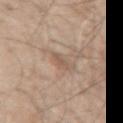Q: Was this lesion biopsied?
A: total-body-photography surveillance lesion; no biopsy
Q: What are the patient's age and sex?
A: male, aged around 50
Q: What kind of image is this?
A: ~15 mm tile from a whole-body skin photo
Q: Lesion location?
A: the right upper arm
Q: How large is the lesion?
A: about 2.5 mm
Q: Illumination type?
A: white-light
Q: Automated lesion metrics?
A: a lesion area of about 3 mm², an outline eccentricity of about 0.85 (0 = round, 1 = elongated), and a shape-asymmetry score of about 0.25 (0 = symmetric); a border-irregularity index near 3/10, a color-variation rating of about 0.5/10, and peripheral color asymmetry of about 0